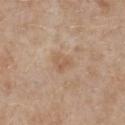Impression: The lesion was tiled from a total-body skin photograph and was not biopsied. Acquisition and patient details: The lesion's longest dimension is about 2.5 mm. Automated image analysis of the tile measured a footprint of about 4.5 mm² and a shape-asymmetry score of about 0.3 (0 = symmetric). Imaged with white-light lighting. Cropped from a total-body skin-imaging series; the visible field is about 15 mm. The patient is a female approximately 40 years of age. Located on the chest.